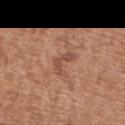The lesion was photographed on a routine skin check and not biopsied; there is no pathology result. From the arm. Imaged with white-light lighting. About 3 mm across. A female subject roughly 65 years of age. An algorithmic analysis of the crop reported a footprint of about 4.5 mm², an outline eccentricity of about 0.8 (0 = round, 1 = elongated), and a symmetry-axis asymmetry near 0.65. And it measured about 8 CIELAB-L* units darker than the surrounding skin and a normalized border contrast of about 6. And it measured a lesion-detection confidence of about 100/100. A close-up tile cropped from a whole-body skin photograph, about 15 mm across.